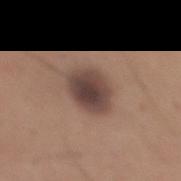Recorded during total-body skin imaging; not selected for excision or biopsy. The tile uses white-light illumination. A male patient, aged approximately 45. The lesion's longest dimension is about 4 mm. An algorithmic analysis of the crop reported border irregularity of about 1.5 on a 0–10 scale. The lesion is on the mid back. A close-up tile cropped from a whole-body skin photograph, about 15 mm across.